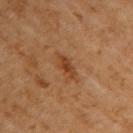This lesion was catalogued during total-body skin photography and was not selected for biopsy. The lesion is located on the right upper arm. A female patient, aged 48 to 52. Automated tile analysis of the lesion measured an outline eccentricity of about 0.9 (0 = round, 1 = elongated) and a symmetry-axis asymmetry near 0.25. The analysis additionally found a border-irregularity index near 3/10 and a color-variation rating of about 2/10. A 15 mm close-up tile from a total-body photography series done for melanoma screening. Approximately 3 mm at its widest.